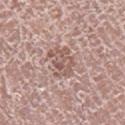follow-up: total-body-photography surveillance lesion; no biopsy
lesion diameter: about 4 mm
tile lighting: white-light illumination
automated metrics: a mean CIELAB color near L≈56 a*≈18 b*≈23, roughly 9 lightness units darker than nearby skin, and a lesion-to-skin contrast of about 6.5 (normalized; higher = more distinct); border irregularity of about 4.5 on a 0–10 scale, a within-lesion color-variation index near 4.5/10, and peripheral color asymmetry of about 1.5
patient: male, in their mid-70s
image: ~15 mm tile from a whole-body skin photo
anatomic site: the right lower leg